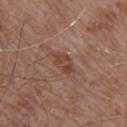Case summary:
* notes · no biopsy performed (imaged during a skin exam)
* location · the upper back
* patient · male, approximately 55 years of age
* image source · total-body-photography crop, ~15 mm field of view